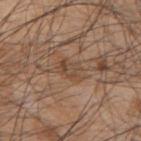Q: Lesion size?
A: ≈3.5 mm
Q: Illumination type?
A: white-light
Q: What did automated image analysis measure?
A: an area of roughly 5 mm² and a shape-asymmetry score of about 0.3 (0 = symmetric); a color-variation rating of about 3.5/10 and a peripheral color-asymmetry measure near 1
Q: What is the imaging modality?
A: ~15 mm crop, total-body skin-cancer survey
Q: Lesion location?
A: the right upper arm
Q: What are the patient's age and sex?
A: male, approximately 45 years of age